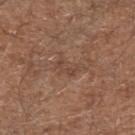{"biopsy_status": "not biopsied; imaged during a skin examination", "lighting": "white-light", "site": "left forearm", "lesion_size": {"long_diameter_mm_approx": 3.0}, "patient": {"sex": "male", "age_approx": 65}, "automated_metrics": {"area_mm2_approx": 4.5, "eccentricity": 0.8, "shape_asymmetry": 0.4, "border_irregularity_0_10": 4.5, "color_variation_0_10": 2.5, "peripheral_color_asymmetry": 1.0, "nevus_likeness_0_100": 0, "lesion_detection_confidence_0_100": 60}, "image": {"source": "total-body photography crop", "field_of_view_mm": 15}}